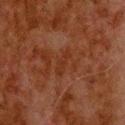<tbp_lesion>
  <biopsy_status>not biopsied; imaged during a skin examination</biopsy_status>
  <image>
    <source>total-body photography crop</source>
    <field_of_view_mm>15</field_of_view_mm>
  </image>
  <site>upper back</site>
  <patient>
    <sex>male</sex>
    <age_approx>80</age_approx>
  </patient>
</tbp_lesion>